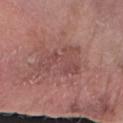Findings:
* workup · no biopsy performed (imaged during a skin exam)
* image-analysis metrics · a lesion color around L≈47 a*≈23 b*≈22 in CIELAB, a lesion–skin lightness drop of about 7, and a normalized border contrast of about 5; an automated nevus-likeness rating near 0 out of 100 and a detector confidence of about 100 out of 100 that the crop contains a lesion
* imaging modality · ~15 mm crop, total-body skin-cancer survey
* subject · female, aged 83 or over
* lesion diameter · about 5.5 mm
* tile lighting · white-light illumination
* anatomic site · the right forearm